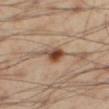Impression: Part of a total-body skin-imaging series; this lesion was reviewed on a skin check and was not flagged for biopsy. Background: Located on the leg. A male patient in their mid-50s. A 15 mm close-up extracted from a 3D total-body photography capture. This is a cross-polarized tile.